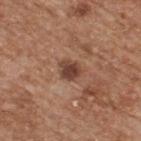Findings:
– biopsy status — catalogued during a skin exam; not biopsied
– patient — male, in their mid- to late 60s
– location — the upper back
– imaging modality — 15 mm crop, total-body photography